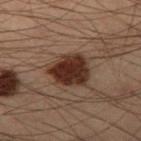<tbp_lesion>
  <biopsy_status>not biopsied; imaged during a skin examination</biopsy_status>
  <lighting>cross-polarized</lighting>
  <image>
    <source>total-body photography crop</source>
    <field_of_view_mm>15</field_of_view_mm>
  </image>
  <patient>
    <sex>male</sex>
    <age_approx>55</age_approx>
  </patient>
  <site>left thigh</site>
  <automated_metrics>
    <cielab_L>24</cielab_L>
    <cielab_a>15</cielab_a>
    <cielab_b>21</cielab_b>
    <vs_skin_darker_L>12.0</vs_skin_darker_L>
    <vs_skin_contrast_norm>13.0</vs_skin_contrast_norm>
  </automated_metrics>
  <lesion_size>
    <long_diameter_mm_approx>4.5</long_diameter_mm_approx>
  </lesion_size>
</tbp_lesion>